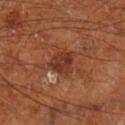No biopsy was performed on this lesion — it was imaged during a full skin examination and was not determined to be concerning. The lesion is on the right lower leg. Captured under cross-polarized illumination. This image is a 15 mm lesion crop taken from a total-body photograph. Measured at roughly 3 mm in maximum diameter. A male subject aged 63 to 67.